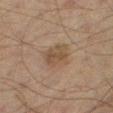Q: Was a biopsy performed?
A: imaged on a skin check; not biopsied
Q: How was the tile lit?
A: cross-polarized
Q: Who is the patient?
A: male, aged approximately 65
Q: What is the anatomic site?
A: the leg
Q: How was this image acquired?
A: total-body-photography crop, ~15 mm field of view
Q: Lesion size?
A: about 3.5 mm